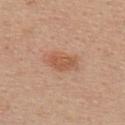| key | value |
|---|---|
| biopsy status | imaged on a skin check; not biopsied |
| illumination | white-light |
| site | the upper back |
| patient | female, about 40 years old |
| acquisition | ~15 mm tile from a whole-body skin photo |
| lesion size | about 4 mm |